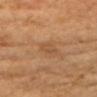| feature | finding |
|---|---|
| biopsy status | no biopsy performed (imaged during a skin exam) |
| image | ~15 mm tile from a whole-body skin photo |
| subject | female, roughly 70 years of age |
| body site | the head or neck |
| automated metrics | border irregularity of about 3 on a 0–10 scale and radial color variation of about 0.5 |
| lighting | cross-polarized |
| lesion diameter | about 2.5 mm |